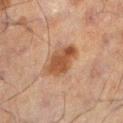{"biopsy_status": "not biopsied; imaged during a skin examination", "image": {"source": "total-body photography crop", "field_of_view_mm": 15}, "site": "left lower leg", "lesion_size": {"long_diameter_mm_approx": 4.5}, "patient": {"sex": "male", "age_approx": 60}, "lighting": "cross-polarized"}